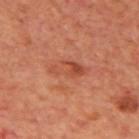Captured during whole-body skin photography for melanoma surveillance; the lesion was not biopsied. The lesion is located on the mid back. About 4.5 mm across. Imaged with cross-polarized lighting. Cropped from a total-body skin-imaging series; the visible field is about 15 mm. A male patient aged around 70.